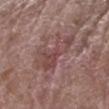{"biopsy_status": "not biopsied; imaged during a skin examination", "patient": {"sex": "female", "age_approx": 70}, "image": {"source": "total-body photography crop", "field_of_view_mm": 15}, "automated_metrics": {"area_mm2_approx": 11.0, "eccentricity": 0.95, "shape_asymmetry": 0.45, "vs_skin_darker_L": 7.0, "vs_skin_contrast_norm": 6.0, "border_irregularity_0_10": 6.0, "color_variation_0_10": 4.0, "peripheral_color_asymmetry": 1.0, "nevus_likeness_0_100": 0, "lesion_detection_confidence_0_100": 90}, "site": "arm", "lesion_size": {"long_diameter_mm_approx": 6.0}, "lighting": "white-light"}